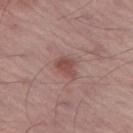biopsy_status: not biopsied; imaged during a skin examination
patient:
  sex: male
  age_approx: 55
lighting: white-light
lesion_size:
  long_diameter_mm_approx: 3.0
site: left thigh
image:
  source: total-body photography crop
  field_of_view_mm: 15